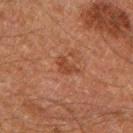The lesion was photographed on a routine skin check and not biopsied; there is no pathology result. On the leg. Measured at roughly 3 mm in maximum diameter. A male patient, about 60 years old. The lesion-visualizer software estimated a footprint of about 3.5 mm², an eccentricity of roughly 0.8, and a symmetry-axis asymmetry near 0.5. The analysis additionally found a mean CIELAB color near L≈33 a*≈21 b*≈27, about 7 CIELAB-L* units darker than the surrounding skin, and a normalized border contrast of about 6.5. The analysis additionally found a nevus-likeness score of about 0/100. Captured under cross-polarized illumination. A close-up tile cropped from a whole-body skin photograph, about 15 mm across.